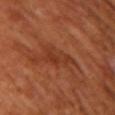notes: imaged on a skin check; not biopsied
lighting: cross-polarized
diameter: about 3.5 mm
image-analysis metrics: an area of roughly 5 mm² and an eccentricity of roughly 0.85
acquisition: 15 mm crop, total-body photography
body site: the right upper arm
subject: female, aged 53 to 57